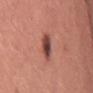Captured during whole-body skin photography for melanoma surveillance; the lesion was not biopsied. About 3.5 mm across. A female subject, roughly 55 years of age. A 15 mm crop from a total-body photograph taken for skin-cancer surveillance. The lesion is on the right thigh.